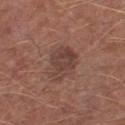<tbp_lesion>
<biopsy_status>not biopsied; imaged during a skin examination</biopsy_status>
<site>leg</site>
<image>
  <source>total-body photography crop</source>
  <field_of_view_mm>15</field_of_view_mm>
</image>
<automated_metrics>
  <eccentricity>0.7</eccentricity>
  <shape_asymmetry>0.3</shape_asymmetry>
  <cielab_L>41</cielab_L>
  <cielab_a>19</cielab_a>
  <cielab_b>23</cielab_b>
  <vs_skin_darker_L>8.0</vs_skin_darker_L>
  <border_irregularity_0_10>3.5</border_irregularity_0_10>
  <peripheral_color_asymmetry>1.0</peripheral_color_asymmetry>
</automated_metrics>
<lesion_size>
  <long_diameter_mm_approx>4.5</long_diameter_mm_approx>
</lesion_size>
<patient>
  <sex>male</sex>
  <age_approx>65</age_approx>
</patient>
<lighting>white-light</lighting>
</tbp_lesion>